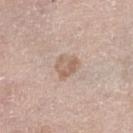Q: Is there a histopathology result?
A: imaged on a skin check; not biopsied
Q: What are the patient's age and sex?
A: female, aged 63–67
Q: What is the imaging modality?
A: 15 mm crop, total-body photography
Q: Where on the body is the lesion?
A: the left lower leg
Q: What lighting was used for the tile?
A: white-light
Q: Lesion size?
A: ~3 mm (longest diameter)